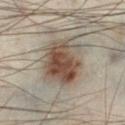Q: Was this lesion biopsied?
A: imaged on a skin check; not biopsied
Q: What lighting was used for the tile?
A: cross-polarized illumination
Q: What is the imaging modality?
A: 15 mm crop, total-body photography
Q: What did automated image analysis measure?
A: a footprint of about 22 mm², an eccentricity of roughly 0.35, and a symmetry-axis asymmetry near 0.15; a color-variation rating of about 6.5/10 and radial color variation of about 2; a nevus-likeness score of about 100/100 and a detector confidence of about 100 out of 100 that the crop contains a lesion
Q: Where on the body is the lesion?
A: the left lower leg
Q: What is the lesion's diameter?
A: ≈5.5 mm
Q: Patient demographics?
A: male, aged 48–52